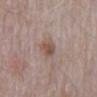Case summary:
• workup — no biopsy performed (imaged during a skin exam)
• image source — ~15 mm crop, total-body skin-cancer survey
• lighting — white-light illumination
• anatomic site — the mid back
• TBP lesion metrics — a border-irregularity rating of about 1.5/10; lesion-presence confidence of about 100/100
• diameter — about 3 mm
• subject — male, roughly 65 years of age